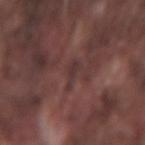This lesion was catalogued during total-body skin photography and was not selected for biopsy. Cropped from a total-body skin-imaging series; the visible field is about 15 mm. The subject is a male aged approximately 75. Longest diameter approximately 3 mm. Located on the left forearm. The total-body-photography lesion software estimated a lesion area of about 3 mm², an outline eccentricity of about 0.9 (0 = round, 1 = elongated), and a symmetry-axis asymmetry near 0.5. The software also gave an average lesion color of about L≈33 a*≈18 b*≈17 (CIELAB) and a lesion–skin lightness drop of about 6. And it measured a within-lesion color-variation index near 0.5/10 and peripheral color asymmetry of about 0.5. The software also gave a nevus-likeness score of about 0/100 and a detector confidence of about 85 out of 100 that the crop contains a lesion. This is a white-light tile.